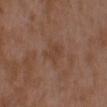Imaged during a routine full-body skin examination; the lesion was not biopsied and no histopathology is available. Cropped from a whole-body photographic skin survey; the tile spans about 15 mm. From the chest. A female patient, approximately 30 years of age.